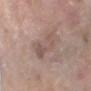biopsy status: total-body-photography surveillance lesion; no biopsy | body site: the arm | lesion diameter: ~5.5 mm (longest diameter) | image source: total-body-photography crop, ~15 mm field of view | tile lighting: white-light | patient: female, approximately 70 years of age | image-analysis metrics: a footprint of about 12 mm², an outline eccentricity of about 0.9 (0 = round, 1 = elongated), and a shape-asymmetry score of about 0.3 (0 = symmetric); a border-irregularity index near 4/10, a color-variation rating of about 4/10, and peripheral color asymmetry of about 1.5; a nevus-likeness score of about 0/100 and a detector confidence of about 95 out of 100 that the crop contains a lesion.